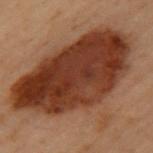Q: Who is the patient?
A: female, roughly 60 years of age
Q: What did automated image analysis measure?
A: an area of roughly 80 mm², a shape eccentricity near 0.75, and a shape-asymmetry score of about 0.3 (0 = symmetric); roughly 14 lightness units darker than nearby skin and a lesion-to-skin contrast of about 12.5 (normalized; higher = more distinct); a border-irregularity index near 4/10 and a color-variation rating of about 7/10; a nevus-likeness score of about 80/100 and lesion-presence confidence of about 100/100
Q: What lighting was used for the tile?
A: cross-polarized illumination
Q: What is the imaging modality?
A: total-body-photography crop, ~15 mm field of view
Q: How large is the lesion?
A: ≈14 mm
Q: Lesion location?
A: the upper back